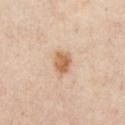workup = total-body-photography surveillance lesion; no biopsy | image = 15 mm crop, total-body photography | subject = aged 53–57 | TBP lesion metrics = border irregularity of about 2 on a 0–10 scale, a color-variation rating of about 3.5/10, and a peripheral color-asymmetry measure near 1.5 | illumination = cross-polarized | body site = the chest.